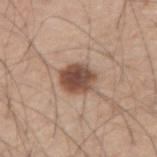biopsy_status: not biopsied; imaged during a skin examination
patient:
  sex: male
  age_approx: 60
automated_metrics:
  area_mm2_approx: 10.0
  shape_asymmetry: 0.2
  vs_skin_darker_L: 16.0
  vs_skin_contrast_norm: 11.0
site: right forearm
lesion_size:
  long_diameter_mm_approx: 4.0
image:
  source: total-body photography crop
  field_of_view_mm: 15
lighting: white-light